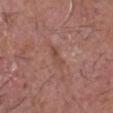A male subject aged around 80. The tile uses white-light illumination. The lesion is on the head or neck. A 15 mm crop from a total-body photograph taken for skin-cancer surveillance.